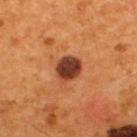biopsy status: catalogued during a skin exam; not biopsied | tile lighting: cross-polarized | subject: male, in their mid-50s | acquisition: ~15 mm crop, total-body skin-cancer survey | location: the upper back | lesion diameter: ≈3 mm.